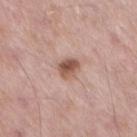| key | value |
|---|---|
| follow-up | no biopsy performed (imaged during a skin exam) |
| acquisition | 15 mm crop, total-body photography |
| body site | the right thigh |
| patient | male, approximately 55 years of age |
| tile lighting | white-light |
| lesion diameter | ~3 mm (longest diameter) |